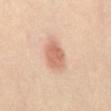subject — female, roughly 40 years of age
anatomic site — the chest
lesion diameter — ~4 mm (longest diameter)
automated metrics — a lesion area of about 9.5 mm² and two-axis asymmetry of about 0.15; a lesion color around L≈65 a*≈22 b*≈32 in CIELAB, about 11 CIELAB-L* units darker than the surrounding skin, and a normalized lesion–skin contrast near 7; a nevus-likeness score of about 100/100 and a lesion-detection confidence of about 100/100
tile lighting — cross-polarized illumination
image source — ~15 mm crop, total-body skin-cancer survey